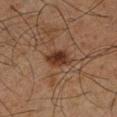Q: Is there a histopathology result?
A: catalogued during a skin exam; not biopsied
Q: What lighting was used for the tile?
A: cross-polarized illumination
Q: How large is the lesion?
A: ≈3.5 mm
Q: What are the patient's age and sex?
A: male, aged approximately 65
Q: Lesion location?
A: the left lower leg
Q: How was this image acquired?
A: ~15 mm tile from a whole-body skin photo
Q: Automated lesion metrics?
A: an area of roughly 6 mm² and two-axis asymmetry of about 0.3; a lesion color around L≈27 a*≈17 b*≈24 in CIELAB and a normalized lesion–skin contrast near 10; an automated nevus-likeness rating near 95 out of 100 and a detector confidence of about 100 out of 100 that the crop contains a lesion